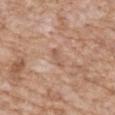Clinical summary: Captured under white-light illumination. The lesion's longest dimension is about 3 mm. The lesion is located on the chest. This image is a 15 mm lesion crop taken from a total-body photograph. A male patient, aged 58 to 62.